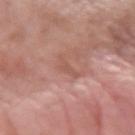{
  "biopsy_status": "not biopsied; imaged during a skin examination",
  "patient": {
    "sex": "female",
    "age_approx": 60
  },
  "site": "left forearm",
  "lighting": "white-light",
  "image": {
    "source": "total-body photography crop",
    "field_of_view_mm": 15
  },
  "lesion_size": {
    "long_diameter_mm_approx": 3.0
  }
}